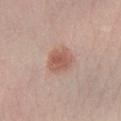Impression:
This lesion was catalogued during total-body skin photography and was not selected for biopsy.
Background:
The lesion is located on the right lower leg. A 15 mm crop from a total-body photograph taken for skin-cancer surveillance. A female subject approximately 45 years of age.